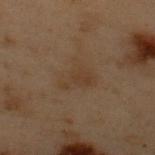Assessment:
This lesion was catalogued during total-body skin photography and was not selected for biopsy.
Image and clinical context:
A male patient, in their mid-50s. Captured under cross-polarized illumination. The recorded lesion diameter is about 3.5 mm. Located on the upper back. A region of skin cropped from a whole-body photographic capture, roughly 15 mm wide.